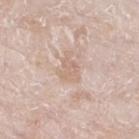| key | value |
|---|---|
| notes | no biopsy performed (imaged during a skin exam) |
| anatomic site | the leg |
| tile lighting | white-light |
| imaging modality | ~15 mm crop, total-body skin-cancer survey |
| diameter | ≈3.5 mm |
| subject | male, approximately 80 years of age |